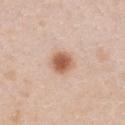A close-up tile cropped from a whole-body skin photograph, about 15 mm across. A female patient, aged 18 to 22. Located on the front of the torso.